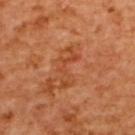The lesion was tiled from a total-body skin photograph and was not biopsied.
The lesion-visualizer software estimated an area of roughly 5.5 mm², a shape eccentricity near 0.95, and two-axis asymmetry of about 0.7. It also reported an automated nevus-likeness rating near 0 out of 100 and a detector confidence of about 100 out of 100 that the crop contains a lesion.
From the back.
The recorded lesion diameter is about 5 mm.
This image is a 15 mm lesion crop taken from a total-body photograph.
A female patient aged 53 to 57.